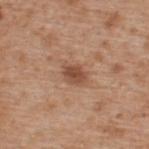Recorded during total-body skin imaging; not selected for excision or biopsy.
A male patient, in their mid- to late 60s.
This is a white-light tile.
A close-up tile cropped from a whole-body skin photograph, about 15 mm across.
Located on the upper back.